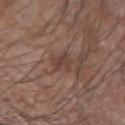Q: Was a biopsy performed?
A: imaged on a skin check; not biopsied
Q: What is the anatomic site?
A: the left forearm
Q: How was this image acquired?
A: 15 mm crop, total-body photography
Q: What are the patient's age and sex?
A: male, aged approximately 75